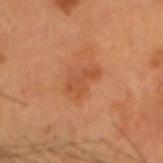Q: Was a biopsy performed?
A: imaged on a skin check; not biopsied
Q: Lesion location?
A: the head or neck
Q: Lesion size?
A: ~3.5 mm (longest diameter)
Q: Who is the patient?
A: male, approximately 55 years of age
Q: What did automated image analysis measure?
A: an average lesion color of about L≈46 a*≈25 b*≈36 (CIELAB) and a lesion–skin lightness drop of about 6; a border-irregularity index near 5.5/10, internal color variation of about 1 on a 0–10 scale, and a peripheral color-asymmetry measure near 0.5; a lesion-detection confidence of about 100/100
Q: How was this image acquired?
A: 15 mm crop, total-body photography
Q: What lighting was used for the tile?
A: cross-polarized illumination